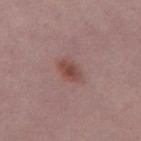{
  "biopsy_status": "not biopsied; imaged during a skin examination",
  "lesion_size": {
    "long_diameter_mm_approx": 3.5
  },
  "patient": {
    "sex": "female",
    "age_approx": 60
  },
  "image": {
    "source": "total-body photography crop",
    "field_of_view_mm": 15
  },
  "site": "left thigh"
}